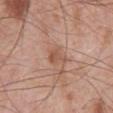Captured during whole-body skin photography for melanoma surveillance; the lesion was not biopsied. The lesion's longest dimension is about 2.5 mm. A male subject approximately 70 years of age. Automated image analysis of the tile measured about 8 CIELAB-L* units darker than the surrounding skin and a normalized lesion–skin contrast near 6. It also reported border irregularity of about 3.5 on a 0–10 scale and peripheral color asymmetry of about 1. A 15 mm close-up extracted from a 3D total-body photography capture. On the front of the torso.